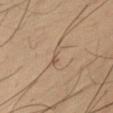Recorded during total-body skin imaging; not selected for excision or biopsy.
About 2.5 mm across.
A male patient, about 50 years old.
On the right upper arm.
A 15 mm close-up extracted from a 3D total-body photography capture.
The tile uses cross-polarized illumination.